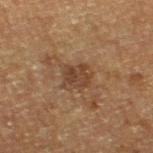site=the leg | subject=male, about 75 years old | acquisition=15 mm crop, total-body photography.